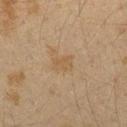Captured during whole-body skin photography for melanoma surveillance; the lesion was not biopsied. Automated tile analysis of the lesion measured a lesion area of about 3.5 mm², an outline eccentricity of about 0.75 (0 = round, 1 = elongated), and two-axis asymmetry of about 0.3. The analysis additionally found a lesion color around L≈44 a*≈12 b*≈29 in CIELAB, a lesion–skin lightness drop of about 5, and a lesion-to-skin contrast of about 5 (normalized; higher = more distinct). It also reported a border-irregularity index near 3/10, a within-lesion color-variation index near 1/10, and a peripheral color-asymmetry measure near 0.5. The lesion is on the left forearm. Measured at roughly 2.5 mm in maximum diameter. This image is a 15 mm lesion crop taken from a total-body photograph. Imaged with cross-polarized lighting. The subject is a female about 40 years old.